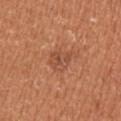  biopsy_status: not biopsied; imaged during a skin examination
  lesion_size:
    long_diameter_mm_approx: 3.5
  patient:
    sex: female
    age_approx: 35
  image:
    source: total-body photography crop
    field_of_view_mm: 15
  lighting: white-light
  automated_metrics:
    border_irregularity_0_10: 2.5
    color_variation_0_10: 4.0
    peripheral_color_asymmetry: 1.5
    nevus_likeness_0_100: 5
  site: arm